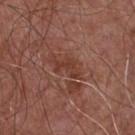Case summary:
* workup · imaged on a skin check; not biopsied
* patient · male, about 55 years old
* site · the chest
* image · ~15 mm crop, total-body skin-cancer survey